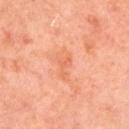workup = total-body-photography surveillance lesion; no biopsy
tile lighting = cross-polarized
image source = ~15 mm crop, total-body skin-cancer survey
diameter = about 2.5 mm
subject = female, approximately 50 years of age
anatomic site = the right upper arm